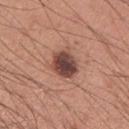Notes:
* image-analysis metrics — a classifier nevus-likeness of about 55/100 and lesion-presence confidence of about 100/100
* tile lighting — white-light illumination
* body site — the arm
* size — ~3.5 mm (longest diameter)
* image source — ~15 mm crop, total-body skin-cancer survey
* patient — male, in their mid- to late 30s